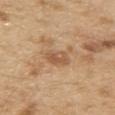Imaged during a routine full-body skin examination; the lesion was not biopsied and no histopathology is available.
The lesion is located on the back.
The total-body-photography lesion software estimated an area of roughly 5 mm², an eccentricity of roughly 0.7, and a symmetry-axis asymmetry near 0.2. It also reported border irregularity of about 2 on a 0–10 scale, internal color variation of about 3 on a 0–10 scale, and radial color variation of about 1. The analysis additionally found a nevus-likeness score of about 0/100 and a detector confidence of about 100 out of 100 that the crop contains a lesion.
Approximately 2.5 mm at its widest.
The patient is a male aged around 70.
Imaged with white-light lighting.
A roughly 15 mm field-of-view crop from a total-body skin photograph.